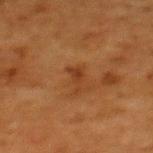Impression: The lesion was tiled from a total-body skin photograph and was not biopsied.